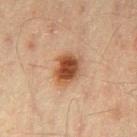Impression:
Captured during whole-body skin photography for melanoma surveillance; the lesion was not biopsied.
Context:
The tile uses cross-polarized illumination. The lesion is located on the left thigh. A male patient aged 43–47. A lesion tile, about 15 mm wide, cut from a 3D total-body photograph. The lesion-visualizer software estimated an average lesion color of about L≈43 a*≈21 b*≈31 (CIELAB) and about 14 CIELAB-L* units darker than the surrounding skin. The analysis additionally found internal color variation of about 5.5 on a 0–10 scale and radial color variation of about 1.5. It also reported a classifier nevus-likeness of about 100/100 and a detector confidence of about 100 out of 100 that the crop contains a lesion.